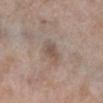Case summary:
- follow-up: catalogued during a skin exam; not biopsied
- subject: female, roughly 75 years of age
- acquisition: 15 mm crop, total-body photography
- automated lesion analysis: a shape eccentricity near 0.7; a nevus-likeness score of about 0/100 and lesion-presence confidence of about 100/100
- site: the right lower leg